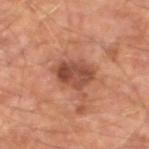The lesion was tiled from a total-body skin photograph and was not biopsied.
A lesion tile, about 15 mm wide, cut from a 3D total-body photograph.
The lesion is located on the left lower leg.
Measured at roughly 4.5 mm in maximum diameter.
Automated tile analysis of the lesion measured an area of roughly 12 mm² and an outline eccentricity of about 0.55 (0 = round, 1 = elongated). The software also gave a nevus-likeness score of about 10/100.
A male subject, aged approximately 65.
This is a cross-polarized tile.